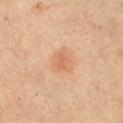notes = imaged on a skin check; not biopsied
illumination = cross-polarized illumination
lesion size = about 2 mm
imaging modality = 15 mm crop, total-body photography
site = the front of the torso
subject = female, aged 43 to 47